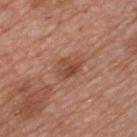<lesion>
  <patient>
    <sex>male</sex>
    <age_approx>50</age_approx>
  </patient>
  <image>
    <source>total-body photography crop</source>
    <field_of_view_mm>15</field_of_view_mm>
  </image>
  <site>chest</site>
  <automated_metrics>
    <area_mm2_approx>5.5</area_mm2_approx>
    <eccentricity>0.7</eccentricity>
    <shape_asymmetry>0.35</shape_asymmetry>
    <border_irregularity_0_10>3.0</border_irregularity_0_10>
    <color_variation_0_10>4.0</color_variation_0_10>
    <peripheral_color_asymmetry>1.5</peripheral_color_asymmetry>
    <nevus_likeness_0_100>50</nevus_likeness_0_100>
    <lesion_detection_confidence_0_100>100</lesion_detection_confidence_0_100>
  </automated_metrics>
  <lighting>white-light</lighting>
  <lesion_size>
    <long_diameter_mm_approx>3.0</long_diameter_mm_approx>
  </lesion_size>
</lesion>